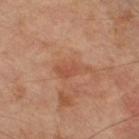Acquisition and patient details:
Captured under cross-polarized illumination. Measured at roughly 4 mm in maximum diameter. A male patient, in their 70s. The lesion-visualizer software estimated a nevus-likeness score of about 0/100. Cropped from a total-body skin-imaging series; the visible field is about 15 mm. On the right thigh.